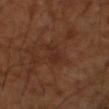Notes:
• notes: imaged on a skin check; not biopsied